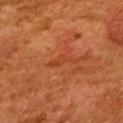Imaged during a routine full-body skin examination; the lesion was not biopsied and no histopathology is available. An algorithmic analysis of the crop reported an average lesion color of about L≈36 a*≈26 b*≈34 (CIELAB), about 5 CIELAB-L* units darker than the surrounding skin, and a lesion-to-skin contrast of about 5 (normalized; higher = more distinct). And it measured a border-irregularity index near 6.5/10, a within-lesion color-variation index near 0/10, and a peripheral color-asymmetry measure near 0. And it measured lesion-presence confidence of about 75/100. A roughly 15 mm field-of-view crop from a total-body skin photograph. Imaged with cross-polarized lighting. The lesion is located on the upper back. The patient is a female aged 48–52.